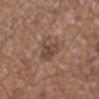The lesion was tiled from a total-body skin photograph and was not biopsied. The lesion is located on the left forearm. The tile uses white-light illumination. The lesion-visualizer software estimated an automated nevus-likeness rating near 0 out of 100 and a lesion-detection confidence of about 100/100. Cropped from a total-body skin-imaging series; the visible field is about 15 mm. A male subject, roughly 55 years of age.